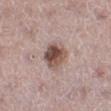<record>
  <biopsy_status>not biopsied; imaged during a skin examination</biopsy_status>
  <automated_metrics>
    <area_mm2_approx>8.5</area_mm2_approx>
    <eccentricity>0.55</eccentricity>
    <cielab_L>50</cielab_L>
    <cielab_a>18</cielab_a>
    <cielab_b>22</cielab_b>
    <vs_skin_darker_L>15.0</vs_skin_darker_L>
    <vs_skin_contrast_norm>10.5</vs_skin_contrast_norm>
    <border_irregularity_0_10>2.0</border_irregularity_0_10>
    <color_variation_0_10>8.0</color_variation_0_10>
    <peripheral_color_asymmetry>3.0</peripheral_color_asymmetry>
  </automated_metrics>
  <patient>
    <sex>female</sex>
    <age_approx>45</age_approx>
  </patient>
  <site>left lower leg</site>
  <lesion_size>
    <long_diameter_mm_approx>3.5</long_diameter_mm_approx>
  </lesion_size>
  <lighting>white-light</lighting>
  <image>
    <source>total-body photography crop</source>
    <field_of_view_mm>15</field_of_view_mm>
  </image>
</record>